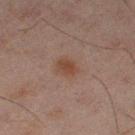Q: Was a biopsy performed?
A: total-body-photography surveillance lesion; no biopsy
Q: Where on the body is the lesion?
A: the left thigh
Q: What are the patient's age and sex?
A: male, aged approximately 45
Q: How was the tile lit?
A: cross-polarized illumination
Q: What did automated image analysis measure?
A: a lesion area of about 4.5 mm² and an eccentricity of roughly 0.6; a lesion color around L≈35 a*≈16 b*≈23 in CIELAB, about 6 CIELAB-L* units darker than the surrounding skin, and a normalized border contrast of about 7; a border-irregularity rating of about 1.5/10, internal color variation of about 2 on a 0–10 scale, and peripheral color asymmetry of about 0.5
Q: How large is the lesion?
A: ≈2.5 mm
Q: How was this image acquired?
A: 15 mm crop, total-body photography